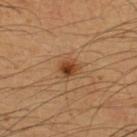| field | value |
|---|---|
| notes | catalogued during a skin exam; not biopsied |
| subject | male, aged around 50 |
| body site | the upper back |
| image | ~15 mm crop, total-body skin-cancer survey |